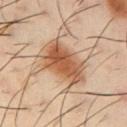The lesion was tiled from a total-body skin photograph and was not biopsied. On the right upper arm. A male subject, aged approximately 40. A 15 mm crop from a total-body photograph taken for skin-cancer surveillance. The tile uses cross-polarized illumination. The recorded lesion diameter is about 6.5 mm.